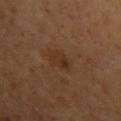{
  "biopsy_status": "not biopsied; imaged during a skin examination",
  "lesion_size": {
    "long_diameter_mm_approx": 2.5
  },
  "site": "left upper arm",
  "patient": {
    "sex": "male",
    "age_approx": 50
  },
  "lighting": "cross-polarized",
  "image": {
    "source": "total-body photography crop",
    "field_of_view_mm": 15
  },
  "automated_metrics": {
    "nevus_likeness_0_100": 15,
    "lesion_detection_confidence_0_100": 100
  }
}